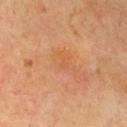Acquisition and patient details: The subject is a female in their mid- to late 50s. Cropped from a whole-body photographic skin survey; the tile spans about 15 mm. The lesion is located on the chest. The lesion-visualizer software estimated an area of roughly 1 mm², a shape eccentricity near 0.8, and two-axis asymmetry of about 0.35. It also reported border irregularity of about 2.5 on a 0–10 scale and a peripheral color-asymmetry measure near 0. And it measured an automated nevus-likeness rating near 0 out of 100 and a lesion-detection confidence of about 100/100.